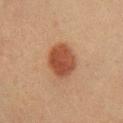imaging modality: 15 mm crop, total-body photography | TBP lesion metrics: an area of roughly 12 mm², an outline eccentricity of about 0.6 (0 = round, 1 = elongated), and a symmetry-axis asymmetry near 0.15; a nevus-likeness score of about 100/100 and a lesion-detection confidence of about 100/100 | lighting: cross-polarized | lesion size: ≈4 mm | body site: the right lower leg | patient: male, aged 43–47.